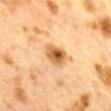workup: total-body-photography surveillance lesion; no biopsy
image source: 15 mm crop, total-body photography
body site: the back
diameter: ~3.5 mm (longest diameter)
patient: female, about 40 years old
tile lighting: cross-polarized illumination